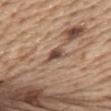Q: Was this lesion biopsied?
A: imaged on a skin check; not biopsied
Q: Where on the body is the lesion?
A: the abdomen
Q: Who is the patient?
A: male, aged 68–72
Q: Illumination type?
A: white-light illumination
Q: What is the lesion's diameter?
A: ~3 mm (longest diameter)
Q: What is the imaging modality?
A: 15 mm crop, total-body photography